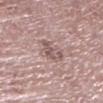lighting=white-light | lesion size=~3.5 mm (longest diameter) | acquisition=~15 mm tile from a whole-body skin photo | location=the left lower leg | patient=male, aged 73 to 77.